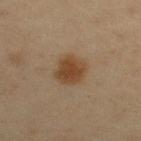The lesion was tiled from a total-body skin photograph and was not biopsied.
Automated tile analysis of the lesion measured roughly 9 lightness units darker than nearby skin.
Longest diameter approximately 3.5 mm.
On the right upper arm.
Imaged with cross-polarized lighting.
A female subject in their mid- to late 40s.
This image is a 15 mm lesion crop taken from a total-body photograph.